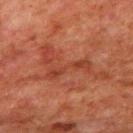Imaged with cross-polarized lighting. A male patient, roughly 80 years of age. Automated image analysis of the tile measured a lesion-to-skin contrast of about 5.5 (normalized; higher = more distinct). And it measured an automated nevus-likeness rating near 0 out of 100 and lesion-presence confidence of about 65/100. On the mid back. A lesion tile, about 15 mm wide, cut from a 3D total-body photograph.